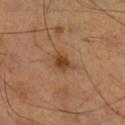Clinical impression:
Recorded during total-body skin imaging; not selected for excision or biopsy.
Clinical summary:
Located on the right lower leg. A region of skin cropped from a whole-body photographic capture, roughly 15 mm wide. Longest diameter approximately 3 mm. This is a cross-polarized tile. A male subject, aged 53–57.